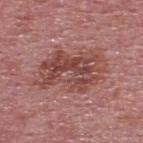The lesion was photographed on a routine skin check and not biopsied; there is no pathology result. The lesion's longest dimension is about 7.5 mm. The lesion is on the upper back. A lesion tile, about 15 mm wide, cut from a 3D total-body photograph. The subject is a male aged 73 to 77.